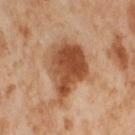Automated image analysis of the tile measured an area of roughly 20 mm², an outline eccentricity of about 0.75 (0 = round, 1 = elongated), and a shape-asymmetry score of about 0.3 (0 = symmetric). And it measured border irregularity of about 3.5 on a 0–10 scale, internal color variation of about 6 on a 0–10 scale, and peripheral color asymmetry of about 2. The software also gave an automated nevus-likeness rating near 65 out of 100 and a detector confidence of about 100 out of 100 that the crop contains a lesion. A 15 mm close-up extracted from a 3D total-body photography capture. The patient is a female approximately 55 years of age. Captured under cross-polarized illumination. From the right thigh. About 7 mm across.